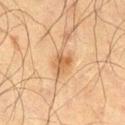| key | value |
|---|---|
| biopsy status | no biopsy performed (imaged during a skin exam) |
| patient | male, in their 70s |
| anatomic site | the right thigh |
| imaging modality | ~15 mm crop, total-body skin-cancer survey |